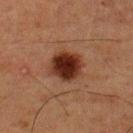Q: Is there a histopathology result?
A: no biopsy performed (imaged during a skin exam)
Q: What did automated image analysis measure?
A: an average lesion color of about L≈24 a*≈20 b*≈23 (CIELAB) and a normalized border contrast of about 14; a color-variation rating of about 4/10 and a peripheral color-asymmetry measure near 1; lesion-presence confidence of about 100/100
Q: What is the anatomic site?
A: the back
Q: Illumination type?
A: cross-polarized
Q: What kind of image is this?
A: ~15 mm tile from a whole-body skin photo
Q: What are the patient's age and sex?
A: male, aged around 60
Q: How large is the lesion?
A: ~4 mm (longest diameter)